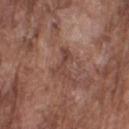biopsy_status: not biopsied; imaged during a skin examination
image:
  source: total-body photography crop
  field_of_view_mm: 15
automated_metrics:
  cielab_L: 43
  cielab_a: 21
  cielab_b: 24
  vs_skin_darker_L: 8.0
  vs_skin_contrast_norm: 6.5
  border_irregularity_0_10: 7.0
  color_variation_0_10: 0.0
  peripheral_color_asymmetry: 0.0
  nevus_likeness_0_100: 0
  lesion_detection_confidence_0_100: 75
lesion_size:
  long_diameter_mm_approx: 3.5
site: right upper arm
patient:
  sex: male
  age_approx: 75
lighting: white-light